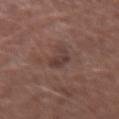Clinical impression:
This lesion was catalogued during total-body skin photography and was not selected for biopsy.
Image and clinical context:
The lesion is located on the right forearm. A region of skin cropped from a whole-body photographic capture, roughly 15 mm wide. The lesion-visualizer software estimated a lesion area of about 4 mm², an outline eccentricity of about 0.55 (0 = round, 1 = elongated), and a symmetry-axis asymmetry near 0.4. And it measured an average lesion color of about L≈37 a*≈18 b*≈19 (CIELAB) and a normalized border contrast of about 7. The software also gave border irregularity of about 4.5 on a 0–10 scale and a peripheral color-asymmetry measure near 0.5. The analysis additionally found a classifier nevus-likeness of about 0/100 and a lesion-detection confidence of about 95/100. The subject is a male aged around 55. The tile uses white-light illumination.